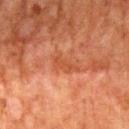Automated tile analysis of the lesion measured a footprint of about 4 mm², an outline eccentricity of about 0.9 (0 = round, 1 = elongated), and a symmetry-axis asymmetry near 0.4. It also reported a mean CIELAB color near L≈40 a*≈26 b*≈33, a lesion–skin lightness drop of about 6, and a lesion-to-skin contrast of about 5.5 (normalized; higher = more distinct). The software also gave border irregularity of about 4.5 on a 0–10 scale, a color-variation rating of about 1/10, and radial color variation of about 0.5. The analysis additionally found a nevus-likeness score of about 0/100 and a lesion-detection confidence of about 100/100. The subject is a male aged around 80. A region of skin cropped from a whole-body photographic capture, roughly 15 mm wide. Approximately 3.5 mm at its widest. The lesion is on the mid back. Captured under cross-polarized illumination.